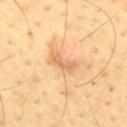notes = no biopsy performed (imaged during a skin exam); patient = male, aged 43–47; body site = the mid back; lighting = cross-polarized illumination; diameter = about 3.5 mm; image = 15 mm crop, total-body photography.